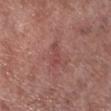No biopsy was performed on this lesion — it was imaged during a full skin examination and was not determined to be concerning. Longest diameter approximately 3 mm. A male patient approximately 55 years of age. Captured under white-light illumination. A region of skin cropped from a whole-body photographic capture, roughly 15 mm wide. The lesion is located on the right lower leg.